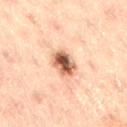Imaged during a routine full-body skin examination; the lesion was not biopsied and no histopathology is available. A male patient, in their mid- to late 40s. About 3.5 mm across. This is a cross-polarized tile. On the leg. Cropped from a whole-body photographic skin survey; the tile spans about 15 mm.